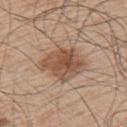The lesion was photographed on a routine skin check and not biopsied; there is no pathology result. The lesion is located on the arm. A 15 mm close-up extracted from a 3D total-body photography capture. About 5.5 mm across. Automated tile analysis of the lesion measured a border-irregularity index near 2.5/10, internal color variation of about 5 on a 0–10 scale, and peripheral color asymmetry of about 1.5. A male subject, aged around 65. This is a white-light tile.